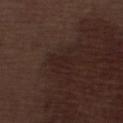workup — total-body-photography surveillance lesion; no biopsy | anatomic site — the left lower leg | subject — male, aged approximately 70 | acquisition — ~15 mm crop, total-body skin-cancer survey.